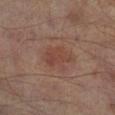Q: Was this lesion biopsied?
A: catalogued during a skin exam; not biopsied
Q: Where on the body is the lesion?
A: the right lower leg
Q: What is the imaging modality?
A: total-body-photography crop, ~15 mm field of view
Q: What is the lesion's diameter?
A: about 4 mm
Q: Who is the patient?
A: male, approximately 65 years of age